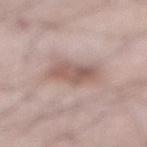No biopsy was performed on this lesion — it was imaged during a full skin examination and was not determined to be concerning. The lesion's longest dimension is about 4 mm. Automated tile analysis of the lesion measured a shape-asymmetry score of about 0.25 (0 = symmetric). The analysis additionally found a detector confidence of about 100 out of 100 that the crop contains a lesion. From the abdomen. A 15 mm close-up extracted from a 3D total-body photography capture. A male patient aged around 55.